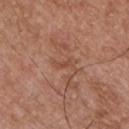Part of a total-body skin-imaging series; this lesion was reviewed on a skin check and was not flagged for biopsy.
A 15 mm crop from a total-body photograph taken for skin-cancer surveillance.
Located on the chest.
A male patient aged 53–57.